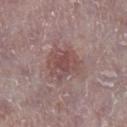Q: Was a biopsy performed?
A: total-body-photography surveillance lesion; no biopsy
Q: Lesion location?
A: the left lower leg
Q: How was the tile lit?
A: white-light illumination
Q: Who is the patient?
A: male, aged 73 to 77
Q: How was this image acquired?
A: ~15 mm tile from a whole-body skin photo